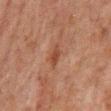Q: Was this lesion biopsied?
A: no biopsy performed (imaged during a skin exam)
Q: How was this image acquired?
A: total-body-photography crop, ~15 mm field of view
Q: Who is the patient?
A: male, aged around 60
Q: Lesion location?
A: the mid back
Q: How large is the lesion?
A: ≈3 mm